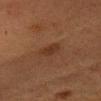  biopsy_status: not biopsied; imaged during a skin examination
  automated_metrics:
    vs_skin_darker_L: 6.0
    vs_skin_contrast_norm: 6.5
    nevus_likeness_0_100: 50
    lesion_detection_confidence_0_100: 100
  site: head or neck
  patient:
    sex: female
    age_approx: 40
  lighting: cross-polarized
  image:
    source: total-body photography crop
    field_of_view_mm: 15